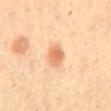biopsy_status: not biopsied; imaged during a skin examination
site: abdomen
automated_metrics:
  cielab_L: 68
  cielab_a: 25
  cielab_b: 37
  vs_skin_darker_L: 12.0
  vs_skin_contrast_norm: 7.0
  border_irregularity_0_10: 1.5
  color_variation_0_10: 3.5
  peripheral_color_asymmetry: 1.0
patient:
  sex: female
  age_approx: 65
lighting: cross-polarized
image:
  source: total-body photography crop
  field_of_view_mm: 15